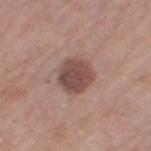Part of a total-body skin-imaging series; this lesion was reviewed on a skin check and was not flagged for biopsy. About 4 mm across. This is a white-light tile. A female patient aged 63–67. A 15 mm close-up extracted from a 3D total-body photography capture. An algorithmic analysis of the crop reported roughly 13 lightness units darker than nearby skin and a lesion-to-skin contrast of about 9.5 (normalized; higher = more distinct). The software also gave a border-irregularity index near 1/10 and a within-lesion color-variation index near 4/10. The lesion is located on the leg.